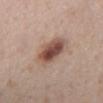Imaged during a routine full-body skin examination; the lesion was not biopsied and no histopathology is available. Located on the mid back. A female patient aged 28 to 32. The lesion-visualizer software estimated a footprint of about 11 mm² and a symmetry-axis asymmetry near 0.15. The analysis additionally found a color-variation rating of about 6.5/10 and peripheral color asymmetry of about 2. The software also gave lesion-presence confidence of about 100/100. The lesion's longest dimension is about 4 mm. This image is a 15 mm lesion crop taken from a total-body photograph. Imaged with white-light lighting.